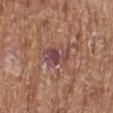Imaged during a routine full-body skin examination; the lesion was not biopsied and no histopathology is available.
Captured under white-light illumination.
Automated tile analysis of the lesion measured a lesion area of about 7.5 mm², an eccentricity of roughly 0.8, and two-axis asymmetry of about 0.25. And it measured a lesion color around L≈45 a*≈23 b*≈20 in CIELAB and a lesion-to-skin contrast of about 9 (normalized; higher = more distinct). The analysis additionally found border irregularity of about 2.5 on a 0–10 scale and radial color variation of about 1.5.
From the left lower leg.
The patient is a female aged approximately 75.
A lesion tile, about 15 mm wide, cut from a 3D total-body photograph.